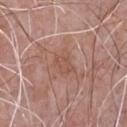Assessment:
Recorded during total-body skin imaging; not selected for excision or biopsy.
Background:
Longest diameter approximately 3 mm. Cropped from a whole-body photographic skin survey; the tile spans about 15 mm. Imaged with white-light lighting. A male subject approximately 70 years of age. The lesion is located on the front of the torso.